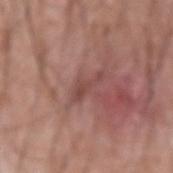Findings:
– follow-up: total-body-photography surveillance lesion; no biopsy
– anatomic site: the left forearm
– patient: male, aged 58 to 62
– image source: ~15 mm crop, total-body skin-cancer survey